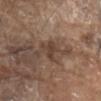Clinical impression: Captured during whole-body skin photography for melanoma surveillance; the lesion was not biopsied. Image and clinical context: Located on the abdomen. The lesion-visualizer software estimated a footprint of about 4 mm², an eccentricity of roughly 0.65, and two-axis asymmetry of about 0.45. The analysis additionally found a peripheral color-asymmetry measure near 0.5. The analysis additionally found a nevus-likeness score of about 0/100 and lesion-presence confidence of about 80/100. A roughly 15 mm field-of-view crop from a total-body skin photograph. The subject is a male aged 78–82. Imaged with white-light lighting.